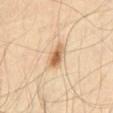workup = no biopsy performed (imaged during a skin exam) | anatomic site = the abdomen | lesion size = about 3.5 mm | tile lighting = cross-polarized | image-analysis metrics = a mean CIELAB color near L≈64 a*≈18 b*≈37 and a normalized border contrast of about 8.5; border irregularity of about 2 on a 0–10 scale, internal color variation of about 7.5 on a 0–10 scale, and peripheral color asymmetry of about 2.5 | patient = male, roughly 65 years of age | acquisition = 15 mm crop, total-body photography.